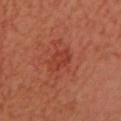– notes — imaged on a skin check; not biopsied
– illumination — cross-polarized
– imaging modality — total-body-photography crop, ~15 mm field of view
– site — the head or neck
– subject — female, aged around 50